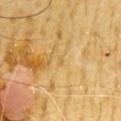A male subject, in their mid-60s. Cropped from a whole-body photographic skin survey; the tile spans about 15 mm. Automated tile analysis of the lesion measured border irregularity of about 2 on a 0–10 scale and peripheral color asymmetry of about 0. The analysis additionally found an automated nevus-likeness rating near 0 out of 100. Longest diameter approximately 1 mm. The lesion is located on the front of the torso. Imaged with cross-polarized lighting.